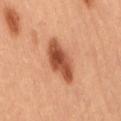workup — total-body-photography surveillance lesion; no biopsy